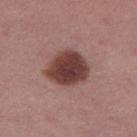follow-up: imaged on a skin check; not biopsied
diameter: ≈5 mm
lighting: white-light
TBP lesion metrics: a footprint of about 16 mm², a shape eccentricity near 0.55, and two-axis asymmetry of about 0.15; a border-irregularity rating of about 1.5/10, internal color variation of about 4 on a 0–10 scale, and peripheral color asymmetry of about 1
image: ~15 mm crop, total-body skin-cancer survey
location: the leg
patient: female, aged approximately 55